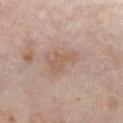No biopsy was performed on this lesion — it was imaged during a full skin examination and was not determined to be concerning.
The total-body-photography lesion software estimated an average lesion color of about L≈58 a*≈18 b*≈29 (CIELAB), about 7 CIELAB-L* units darker than the surrounding skin, and a normalized border contrast of about 5.5. The analysis additionally found border irregularity of about 5 on a 0–10 scale, a within-lesion color-variation index near 1/10, and peripheral color asymmetry of about 0.5.
Cropped from a whole-body photographic skin survey; the tile spans about 15 mm.
The tile uses white-light illumination.
About 4 mm across.
A female subject aged 63 to 67.
Located on the chest.